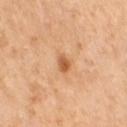Captured during whole-body skin photography for melanoma surveillance; the lesion was not biopsied. The recorded lesion diameter is about 2.5 mm. A female subject, about 55 years old. Cropped from a total-body skin-imaging series; the visible field is about 15 mm. From the right thigh. The tile uses cross-polarized illumination.